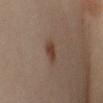Assessment: The lesion was photographed on a routine skin check and not biopsied; there is no pathology result. Image and clinical context: Longest diameter approximately 2.5 mm. On the left arm. A 15 mm close-up extracted from a 3D total-body photography capture. A female patient, aged 38 to 42. Automated image analysis of the tile measured a footprint of about 3.5 mm², a shape eccentricity near 0.8, and two-axis asymmetry of about 0.35. Captured under cross-polarized illumination.